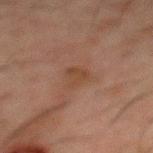<tbp_lesion>
<biopsy_status>not biopsied; imaged during a skin examination</biopsy_status>
<automated_metrics>
  <cielab_L>33</cielab_L>
  <cielab_a>16</cielab_a>
  <cielab_b>24</cielab_b>
  <vs_skin_darker_L>5.0</vs_skin_darker_L>
  <vs_skin_contrast_norm>6.0</vs_skin_contrast_norm>
  <border_irregularity_0_10>3.5</border_irregularity_0_10>
  <color_variation_0_10>2.0</color_variation_0_10>
</automated_metrics>
<site>mid back</site>
<image>
  <source>total-body photography crop</source>
  <field_of_view_mm>15</field_of_view_mm>
</image>
<lighting>cross-polarized</lighting>
<lesion_size>
  <long_diameter_mm_approx>2.5</long_diameter_mm_approx>
</lesion_size>
<patient>
  <sex>male</sex>
  <age_approx>50</age_approx>
</patient>
</tbp_lesion>